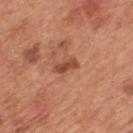biopsy status: imaged on a skin check; not biopsied | lesion diameter: ~3 mm (longest diameter) | image source: ~15 mm tile from a whole-body skin photo | location: the mid back | automated lesion analysis: an area of roughly 3.5 mm² and an outline eccentricity of about 0.9 (0 = round, 1 = elongated); an average lesion color of about L≈48 a*≈26 b*≈31 (CIELAB) and a lesion–skin lightness drop of about 11; an automated nevus-likeness rating near 35 out of 100 and a detector confidence of about 100 out of 100 that the crop contains a lesion | patient: male, about 65 years old | tile lighting: white-light.